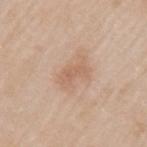The lesion was photographed on a routine skin check and not biopsied; there is no pathology result. This image is a 15 mm lesion crop taken from a total-body photograph. Approximately 3 mm at its widest. Automated image analysis of the tile measured a footprint of about 3 mm² and a symmetry-axis asymmetry near 0.35. And it measured a lesion color around L≈61 a*≈20 b*≈31 in CIELAB, a lesion–skin lightness drop of about 7, and a normalized lesion–skin contrast near 5. The software also gave a nevus-likeness score of about 10/100 and a detector confidence of about 100 out of 100 that the crop contains a lesion. Imaged with white-light lighting. A female subject, in their mid-50s. Located on the left upper arm.